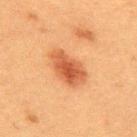Recorded during total-body skin imaging; not selected for excision or biopsy. Longest diameter approximately 5 mm. A 15 mm close-up extracted from a 3D total-body photography capture. A male subject aged around 50. An algorithmic analysis of the crop reported a footprint of about 10 mm², an eccentricity of roughly 0.85, and a symmetry-axis asymmetry near 0.2. It also reported a lesion–skin lightness drop of about 11 and a lesion-to-skin contrast of about 8.5 (normalized; higher = more distinct). The software also gave a nevus-likeness score of about 95/100 and a lesion-detection confidence of about 100/100. Located on the upper back.